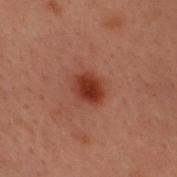<tbp_lesion>
<biopsy_status>not biopsied; imaged during a skin examination</biopsy_status>
<patient>
  <sex>male</sex>
  <age_approx>30</age_approx>
</patient>
<site>head or neck</site>
<image>
  <source>total-body photography crop</source>
  <field_of_view_mm>15</field_of_view_mm>
</image>
<lighting>cross-polarized</lighting>
<lesion_size>
  <long_diameter_mm_approx>3.0</long_diameter_mm_approx>
</lesion_size>
<automated_metrics>
  <area_mm2_approx>6.0</area_mm2_approx>
  <eccentricity>0.6</eccentricity>
  <shape_asymmetry>0.2</shape_asymmetry>
</automated_metrics>
</tbp_lesion>